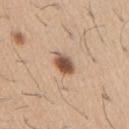subject — male, in their 60s
image — ~15 mm crop, total-body skin-cancer survey
tile lighting — white-light
body site — the right upper arm